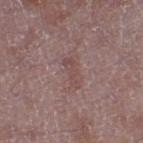Case summary:
• workup · no biopsy performed (imaged during a skin exam)
• size · about 3.5 mm
• imaging modality · ~15 mm tile from a whole-body skin photo
• TBP lesion metrics · a border-irregularity rating of about 5/10, a color-variation rating of about 1/10, and a peripheral color-asymmetry measure near 0
• location · the left thigh
• patient · male, aged around 50